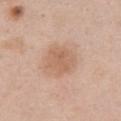Q: Was a biopsy performed?
A: no biopsy performed (imaged during a skin exam)
Q: How was this image acquired?
A: ~15 mm crop, total-body skin-cancer survey
Q: Lesion location?
A: the abdomen
Q: What is the lesion's diameter?
A: ≈3.5 mm
Q: Patient demographics?
A: female, in their 50s
Q: Illumination type?
A: white-light illumination